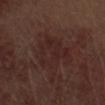This lesion was catalogued during total-body skin photography and was not selected for biopsy. A male subject, approximately 70 years of age. An algorithmic analysis of the crop reported an automated nevus-likeness rating near 0 out of 100 and a detector confidence of about 100 out of 100 that the crop contains a lesion. On the right thigh. This is a white-light tile. A roughly 15 mm field-of-view crop from a total-body skin photograph. Longest diameter approximately 5 mm.